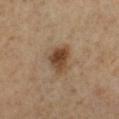{
  "biopsy_status": "not biopsied; imaged during a skin examination",
  "lesion_size": {
    "long_diameter_mm_approx": 3.5
  },
  "patient": {
    "sex": "male",
    "age_approx": 65
  },
  "automated_metrics": {
    "nevus_likeness_0_100": 95,
    "lesion_detection_confidence_0_100": 100
  },
  "site": "right lower leg",
  "lighting": "cross-polarized",
  "image": {
    "source": "total-body photography crop",
    "field_of_view_mm": 15
  }
}